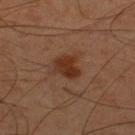Assessment: Recorded during total-body skin imaging; not selected for excision or biopsy. Clinical summary: Captured under cross-polarized illumination. A lesion tile, about 15 mm wide, cut from a 3D total-body photograph. A male patient, aged 43–47. The total-body-photography lesion software estimated a normalized lesion–skin contrast near 9. The software also gave a border-irregularity index near 3.5/10 and peripheral color asymmetry of about 0.5. And it measured an automated nevus-likeness rating near 80 out of 100 and lesion-presence confidence of about 100/100. The recorded lesion diameter is about 3 mm. Located on the upper back.